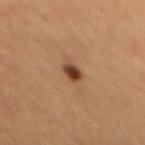Assessment: The lesion was photographed on a routine skin check and not biopsied; there is no pathology result. Clinical summary: Longest diameter approximately 2.5 mm. The lesion-visualizer software estimated an eccentricity of roughly 0.7 and a shape-asymmetry score of about 0.25 (0 = symmetric). And it measured a lesion color around L≈37 a*≈20 b*≈29 in CIELAB, a lesion–skin lightness drop of about 13, and a normalized lesion–skin contrast near 10.5. And it measured a border-irregularity rating of about 2/10, a within-lesion color-variation index near 4/10, and peripheral color asymmetry of about 1.5. On the back. Cropped from a total-body skin-imaging series; the visible field is about 15 mm. A male patient, aged 58 to 62. The tile uses cross-polarized illumination.